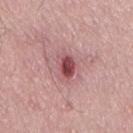- follow-up · total-body-photography surveillance lesion; no biopsy
- automated metrics · an area of roughly 7.5 mm², a shape eccentricity near 0.7, and two-axis asymmetry of about 0.25; a mean CIELAB color near L≈51 a*≈27 b*≈19, roughly 13 lightness units darker than nearby skin, and a normalized lesion–skin contrast near 8.5; a nevus-likeness score of about 45/100 and lesion-presence confidence of about 100/100
- patient · male, about 50 years old
- site · the lower back
- lesion diameter · ~4 mm (longest diameter)
- tile lighting · white-light
- acquisition · total-body-photography crop, ~15 mm field of view